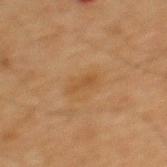Case summary:
- notes: imaged on a skin check; not biopsied
- subject: male, aged approximately 65
- image source: ~15 mm tile from a whole-body skin photo
- automated metrics: an area of roughly 2.5 mm², an outline eccentricity of about 0.9 (0 = round, 1 = elongated), and a shape-asymmetry score of about 0.3 (0 = symmetric); a mean CIELAB color near L≈41 a*≈17 b*≈34, a lesion–skin lightness drop of about 5, and a normalized border contrast of about 5.5; peripheral color asymmetry of about 0
- illumination: cross-polarized illumination
- anatomic site: the mid back